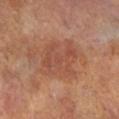Findings:
* workup · imaged on a skin check; not biopsied
* subject · female, roughly 75 years of age
* imaging modality · total-body-photography crop, ~15 mm field of view
* body site · the left lower leg
* image-analysis metrics · about 7 CIELAB-L* units darker than the surrounding skin and a normalized lesion–skin contrast near 5; a border-irregularity index near 5.5/10; a nevus-likeness score of about 0/100
* lighting · cross-polarized illumination